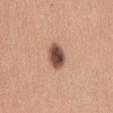Q: Is there a histopathology result?
A: no biopsy performed (imaged during a skin exam)
Q: Who is the patient?
A: female, aged approximately 30
Q: Automated lesion metrics?
A: an area of roughly 6.5 mm² and a shape eccentricity near 0.75; a mean CIELAB color near L≈50 a*≈21 b*≈27, roughly 19 lightness units darker than nearby skin, and a normalized border contrast of about 12.5
Q: What kind of image is this?
A: ~15 mm crop, total-body skin-cancer survey
Q: How large is the lesion?
A: ~3.5 mm (longest diameter)
Q: What is the anatomic site?
A: the front of the torso
Q: What lighting was used for the tile?
A: white-light